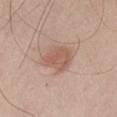Q: Was this lesion biopsied?
A: total-body-photography surveillance lesion; no biopsy
Q: Patient demographics?
A: male, aged 48 to 52
Q: What is the lesion's diameter?
A: ≈4 mm
Q: How was the tile lit?
A: white-light
Q: Where on the body is the lesion?
A: the chest
Q: How was this image acquired?
A: ~15 mm crop, total-body skin-cancer survey